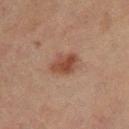A 15 mm close-up tile from a total-body photography series done for melanoma screening.
A female subject about 55 years old.
The lesion is on the right thigh.
Captured under cross-polarized illumination.
About 3.5 mm across.
An algorithmic analysis of the crop reported a mean CIELAB color near L≈41 a*≈20 b*≈26 and a normalized lesion–skin contrast near 8. And it measured a border-irregularity rating of about 2/10, internal color variation of about 4 on a 0–10 scale, and peripheral color asymmetry of about 1.5. It also reported an automated nevus-likeness rating near 85 out of 100 and lesion-presence confidence of about 100/100.